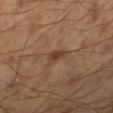Assessment: Imaged during a routine full-body skin examination; the lesion was not biopsied and no histopathology is available. Image and clinical context: A male subject about 45 years old. Measured at roughly 3 mm in maximum diameter. The lesion is on the left lower leg. A 15 mm close-up tile from a total-body photography series done for melanoma screening.